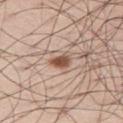Q: Was this lesion biopsied?
A: total-body-photography surveillance lesion; no biopsy